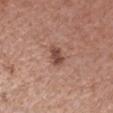  site: right forearm
  image:
    source: total-body photography crop
    field_of_view_mm: 15
  lesion_size:
    long_diameter_mm_approx: 2.5
  patient:
    sex: female
    age_approx: 40
  lighting: white-light
  automated_metrics:
    cielab_L: 47
    cielab_a: 22
    cielab_b: 26
    vs_skin_darker_L: 12.0
    vs_skin_contrast_norm: 8.5
    border_irregularity_0_10: 2.5
    peripheral_color_asymmetry: 0.5
    nevus_likeness_0_100: 75
    lesion_detection_confidence_0_100: 100